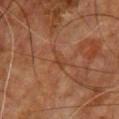Notes:
* follow-up · catalogued during a skin exam; not biopsied
* anatomic site · the chest
* image · ~15 mm crop, total-body skin-cancer survey
* subject · male, aged around 60